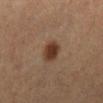The lesion was photographed on a routine skin check and not biopsied; there is no pathology result. This is a cross-polarized tile. Measured at roughly 3 mm in maximum diameter. From the right lower leg. The subject is a female about 60 years old. A region of skin cropped from a whole-body photographic capture, roughly 15 mm wide.